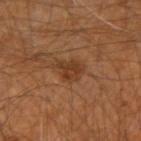The lesion was tiled from a total-body skin photograph and was not biopsied. The lesion-visualizer software estimated a classifier nevus-likeness of about 20/100 and lesion-presence confidence of about 100/100. A close-up tile cropped from a whole-body skin photograph, about 15 mm across. The tile uses cross-polarized illumination. The recorded lesion diameter is about 3.5 mm. The lesion is on the arm. A male patient, aged 58–62.